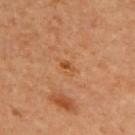From the upper back. The recorded lesion diameter is about 2.5 mm. A 15 mm close-up tile from a total-body photography series done for melanoma screening. A female patient, approximately 55 years of age. This is a cross-polarized tile.